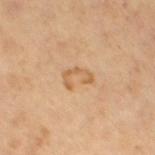The lesion was photographed on a routine skin check and not biopsied; there is no pathology result.
From the left thigh.
The lesion-visualizer software estimated an average lesion color of about L≈60 a*≈19 b*≈38 (CIELAB) and a normalized border contrast of about 6. The software also gave a classifier nevus-likeness of about 0/100 and lesion-presence confidence of about 100/100.
The lesion's longest dimension is about 3 mm.
A region of skin cropped from a whole-body photographic capture, roughly 15 mm wide.
A female patient, aged approximately 65.
Captured under cross-polarized illumination.